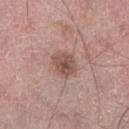{"biopsy_status": "not biopsied; imaged during a skin examination", "site": "right lower leg", "patient": {"sex": "male", "age_approx": 65}, "image": {"source": "total-body photography crop", "field_of_view_mm": 15}}